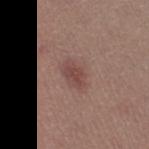No biopsy was performed on this lesion — it was imaged during a full skin examination and was not determined to be concerning. A female subject roughly 20 years of age. A lesion tile, about 15 mm wide, cut from a 3D total-body photograph. The lesion is on the right thigh.